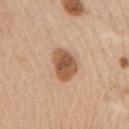The lesion was tiled from a total-body skin photograph and was not biopsied. Measured at roughly 4 mm in maximum diameter. This is a white-light tile. An algorithmic analysis of the crop reported a footprint of about 9.5 mm². The lesion is located on the chest. A female subject aged around 70. A 15 mm crop from a total-body photograph taken for skin-cancer surveillance.